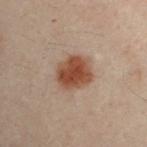Q: Was this lesion biopsied?
A: total-body-photography surveillance lesion; no biopsy
Q: Illumination type?
A: cross-polarized illumination
Q: How large is the lesion?
A: ~4.5 mm (longest diameter)
Q: What kind of image is this?
A: 15 mm crop, total-body photography
Q: What are the patient's age and sex?
A: male, aged 28–32
Q: Lesion location?
A: the arm